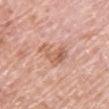The lesion was photographed on a routine skin check and not biopsied; there is no pathology result.
Automated tile analysis of the lesion measured a nevus-likeness score of about 0/100 and lesion-presence confidence of about 100/100.
Captured under white-light illumination.
The recorded lesion diameter is about 4 mm.
A female patient aged approximately 65.
The lesion is on the chest.
A region of skin cropped from a whole-body photographic capture, roughly 15 mm wide.